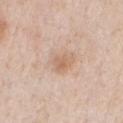<tbp_lesion>
<biopsy_status>not biopsied; imaged during a skin examination</biopsy_status>
<lesion_size>
  <long_diameter_mm_approx>3.5</long_diameter_mm_approx>
</lesion_size>
<patient>
  <sex>male</sex>
  <age_approx>60</age_approx>
</patient>
<automated_metrics>
  <area_mm2_approx>9.5</area_mm2_approx>
  <eccentricity>0.45</eccentricity>
  <shape_asymmetry>0.15</shape_asymmetry>
  <border_irregularity_0_10>2.0</border_irregularity_0_10>
  <color_variation_0_10>3.5</color_variation_0_10>
  <peripheral_color_asymmetry>1.0</peripheral_color_asymmetry>
  <nevus_likeness_0_100>5</nevus_likeness_0_100>
  <lesion_detection_confidence_0_100>100</lesion_detection_confidence_0_100>
</automated_metrics>
<site>chest</site>
<image>
  <source>total-body photography crop</source>
  <field_of_view_mm>15</field_of_view_mm>
</image>
</tbp_lesion>